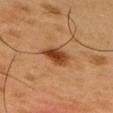Clinical summary:
Automated image analysis of the tile measured a footprint of about 6 mm², an eccentricity of roughly 0.65, and two-axis asymmetry of about 0.25. And it measured a classifier nevus-likeness of about 100/100 and lesion-presence confidence of about 100/100. Longest diameter approximately 3 mm. A male subject, aged 48–52. The lesion is located on the upper back. Captured under cross-polarized illumination. Cropped from a total-body skin-imaging series; the visible field is about 15 mm.